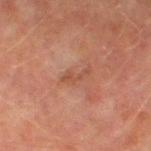workup = imaged on a skin check; not biopsied
body site = the left thigh
image source = ~15 mm crop, total-body skin-cancer survey
subject = male, in their mid- to late 70s
illumination = cross-polarized
size = ≈3.5 mm